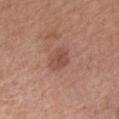Clinical impression:
No biopsy was performed on this lesion — it was imaged during a full skin examination and was not determined to be concerning.
Background:
This is a white-light tile. A 15 mm close-up tile from a total-body photography series done for melanoma screening. A female patient approximately 60 years of age. Measured at roughly 2.5 mm in maximum diameter. The lesion is on the arm.